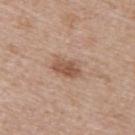Recorded during total-body skin imaging; not selected for excision or biopsy.
The lesion is located on the upper back.
A female subject, about 40 years old.
A close-up tile cropped from a whole-body skin photograph, about 15 mm across.